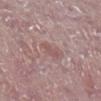The subject is a male aged 73 to 77. From the right lower leg. This is a white-light tile. A 15 mm crop from a total-body photograph taken for skin-cancer surveillance.